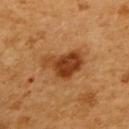Clinical impression: Part of a total-body skin-imaging series; this lesion was reviewed on a skin check and was not flagged for biopsy. Image and clinical context: The subject is a female in their mid- to late 50s. Captured under cross-polarized illumination. The lesion is on the back. Approximately 5 mm at its widest. A 15 mm crop from a total-body photograph taken for skin-cancer surveillance.